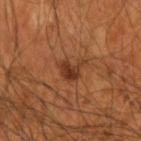Context:
The lesion is located on the right forearm. The recorded lesion diameter is about 3 mm. A male patient approximately 55 years of age. Cropped from a total-body skin-imaging series; the visible field is about 15 mm.